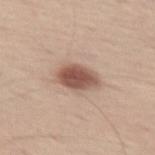Imaged with white-light lighting.
On the abdomen.
A lesion tile, about 15 mm wide, cut from a 3D total-body photograph.
A male patient, in their mid-70s.